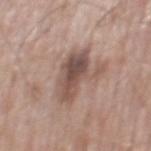  biopsy_status: not biopsied; imaged during a skin examination
  patient:
    sex: male
    age_approx: 75
  lighting: white-light
  site: back
  image:
    source: total-body photography crop
    field_of_view_mm: 15
  lesion_size:
    long_diameter_mm_approx: 5.5
  automated_metrics:
    area_mm2_approx: 15.0
    eccentricity: 0.75
    cielab_L: 51
    cielab_a: 17
    cielab_b: 23
    vs_skin_darker_L: 12.0
    nevus_likeness_0_100: 10
    lesion_detection_confidence_0_100: 100